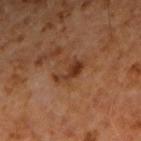Assessment:
Recorded during total-body skin imaging; not selected for excision or biopsy.
Acquisition and patient details:
An algorithmic analysis of the crop reported a mean CIELAB color near L≈34 a*≈21 b*≈30, a lesion–skin lightness drop of about 9, and a normalized border contrast of about 8. It also reported an automated nevus-likeness rating near 20 out of 100 and lesion-presence confidence of about 100/100. Imaged with cross-polarized lighting. A 15 mm close-up tile from a total-body photography series done for melanoma screening. About 3.5 mm across. On the right forearm. A male patient, aged 58 to 62.